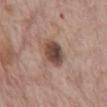This lesion was catalogued during total-body skin photography and was not selected for biopsy.
The lesion-visualizer software estimated a lesion area of about 9 mm² and a symmetry-axis asymmetry near 0.15. It also reported border irregularity of about 1.5 on a 0–10 scale, a within-lesion color-variation index near 6/10, and radial color variation of about 1.5.
Longest diameter approximately 4 mm.
The lesion is on the abdomen.
Cropped from a total-body skin-imaging series; the visible field is about 15 mm.
Captured under white-light illumination.
A male patient, in their 70s.